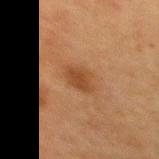Clinical impression:
Recorded during total-body skin imaging; not selected for excision or biopsy.